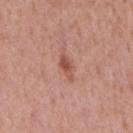Imaged during a routine full-body skin examination; the lesion was not biopsied and no histopathology is available.
Captured under white-light illumination.
An algorithmic analysis of the crop reported an outline eccentricity of about 0.9 (0 = round, 1 = elongated). It also reported a nevus-likeness score of about 65/100 and a lesion-detection confidence of about 100/100.
This image is a 15 mm lesion crop taken from a total-body photograph.
A male patient, aged around 55.
The lesion is located on the mid back.